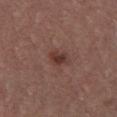Context:
A male patient, aged around 40. A lesion tile, about 15 mm wide, cut from a 3D total-body photograph. Located on the chest.